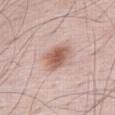Captured during whole-body skin photography for melanoma surveillance; the lesion was not biopsied. A 15 mm close-up extracted from a 3D total-body photography capture. The subject is a male in their mid-60s. This is a white-light tile. On the abdomen.